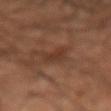notes = catalogued during a skin exam; not biopsied
size = about 3 mm
lighting = cross-polarized illumination
anatomic site = the right forearm
image source = ~15 mm tile from a whole-body skin photo
patient = male, aged around 45